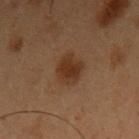Case summary:
• biopsy status · total-body-photography surveillance lesion; no biopsy
• lighting · cross-polarized illumination
• patient · male, in their mid- to late 50s
• body site · the arm
• lesion size · ≈3.5 mm
• imaging modality · ~15 mm tile from a whole-body skin photo